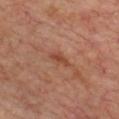Clinical impression:
Captured during whole-body skin photography for melanoma surveillance; the lesion was not biopsied.
Context:
A male subject in their 70s. Cropped from a total-body skin-imaging series; the visible field is about 15 mm. Located on the chest.